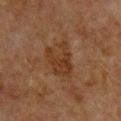Clinical impression: Captured during whole-body skin photography for melanoma surveillance; the lesion was not biopsied. Background: Approximately 4.5 mm at its widest. From the front of the torso. A 15 mm crop from a total-body photograph taken for skin-cancer surveillance. A male patient, approximately 50 years of age.